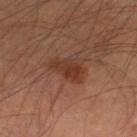Captured during whole-body skin photography for melanoma surveillance; the lesion was not biopsied.
A male subject, about 65 years old.
Automated tile analysis of the lesion measured a lesion area of about 9 mm², an outline eccentricity of about 0.75 (0 = round, 1 = elongated), and a symmetry-axis asymmetry near 0.4.
Approximately 4.5 mm at its widest.
Located on the right lower leg.
A 15 mm close-up tile from a total-body photography series done for melanoma screening.